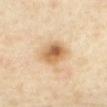Notes:
• notes — no biopsy performed (imaged during a skin exam)
• lesion size — ~4 mm (longest diameter)
• site — the mid back
• lighting — cross-polarized illumination
• patient — female, roughly 40 years of age
• image-analysis metrics — an area of roughly 9 mm², an outline eccentricity of about 0.7 (0 = round, 1 = elongated), and a shape-asymmetry score of about 0.2 (0 = symmetric); a within-lesion color-variation index near 7.5/10 and peripheral color asymmetry of about 2.5
• acquisition — 15 mm crop, total-body photography